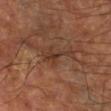follow-up: imaged on a skin check; not biopsied | lesion diameter: ~5 mm (longest diameter) | patient: male, aged around 60 | illumination: cross-polarized illumination | image source: ~15 mm crop, total-body skin-cancer survey | image-analysis metrics: a lesion color around L≈35 a*≈19 b*≈27 in CIELAB, a lesion–skin lightness drop of about 7, and a normalized lesion–skin contrast near 6.5; a lesion-detection confidence of about 85/100 | location: the leg.